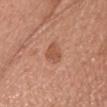Q: Was this lesion biopsied?
A: imaged on a skin check; not biopsied
Q: What are the patient's age and sex?
A: female, in their mid- to late 60s
Q: How was the tile lit?
A: white-light
Q: What is the imaging modality?
A: ~15 mm crop, total-body skin-cancer survey
Q: Lesion size?
A: ~3 mm (longest diameter)
Q: Lesion location?
A: the chest